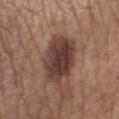biopsy_status: not biopsied; imaged during a skin examination
lighting: white-light
lesion_size:
  long_diameter_mm_approx: 5.5
site: left forearm
image:
  source: total-body photography crop
  field_of_view_mm: 15
patient:
  sex: male
  age_approx: 50